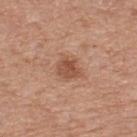A region of skin cropped from a whole-body photographic capture, roughly 15 mm wide. A male patient, roughly 80 years of age. Located on the upper back.